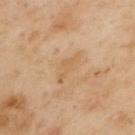| feature | finding |
|---|---|
| workup | catalogued during a skin exam; not biopsied |
| tile lighting | cross-polarized |
| lesion diameter | about 4 mm |
| patient | male, roughly 55 years of age |
| body site | the left upper arm |
| image | ~15 mm crop, total-body skin-cancer survey |
| automated metrics | a lesion area of about 4 mm² and an outline eccentricity of about 0.95 (0 = round, 1 = elongated); a lesion color around L≈61 a*≈18 b*≈38 in CIELAB; radial color variation of about 0; a classifier nevus-likeness of about 0/100 and a lesion-detection confidence of about 100/100 |